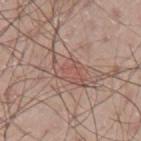Assessment: The lesion was photographed on a routine skin check and not biopsied; there is no pathology result. Image and clinical context: The subject is a male about 65 years old. The lesion is on the left upper arm. A lesion tile, about 15 mm wide, cut from a 3D total-body photograph.